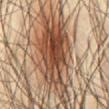This lesion was catalogued during total-body skin photography and was not selected for biopsy. Imaged with cross-polarized lighting. The lesion is on the abdomen. About 10.5 mm across. A region of skin cropped from a whole-body photographic capture, roughly 15 mm wide. The subject is a male roughly 65 years of age.